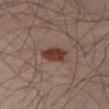The lesion was photographed on a routine skin check and not biopsied; there is no pathology result. Automated tile analysis of the lesion measured an outline eccentricity of about 0.75 (0 = round, 1 = elongated). And it measured a border-irregularity rating of about 2/10 and a peripheral color-asymmetry measure near 1. From the right thigh. This is a cross-polarized tile. The subject is a male about 65 years old. A 15 mm crop from a total-body photograph taken for skin-cancer surveillance. About 3.5 mm across.